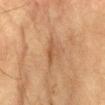{
  "biopsy_status": "not biopsied; imaged during a skin examination",
  "lighting": "cross-polarized",
  "patient": {
    "sex": "male",
    "age_approx": "85+"
  },
  "image": {
    "source": "total-body photography crop",
    "field_of_view_mm": 15
  },
  "site": "front of the torso"
}